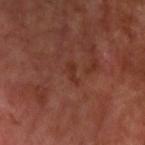The lesion was tiled from a total-body skin photograph and was not biopsied.
A region of skin cropped from a whole-body photographic capture, roughly 15 mm wide.
A male subject roughly 65 years of age.
The tile uses cross-polarized illumination.
The lesion is on the left upper arm.
The lesion-visualizer software estimated a lesion area of about 2 mm² and a shape eccentricity near 0.9. It also reported a classifier nevus-likeness of about 0/100 and a detector confidence of about 100 out of 100 that the crop contains a lesion.